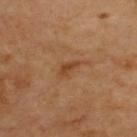notes = no biopsy performed (imaged during a skin exam)
image-analysis metrics = an average lesion color of about L≈42 a*≈23 b*≈35 (CIELAB), about 8 CIELAB-L* units darker than the surrounding skin, and a normalized border contrast of about 7; a classifier nevus-likeness of about 45/100 and a detector confidence of about 100 out of 100 that the crop contains a lesion
image = 15 mm crop, total-body photography
body site = the upper back
size = ≈2.5 mm
subject = roughly 65 years of age
tile lighting = cross-polarized